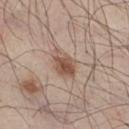{"biopsy_status": "not biopsied; imaged during a skin examination", "lighting": "white-light", "lesion_size": {"long_diameter_mm_approx": 3.5}, "image": {"source": "total-body photography crop", "field_of_view_mm": 15}, "site": "right thigh", "automated_metrics": {"area_mm2_approx": 7.0, "eccentricity": 0.7}, "patient": {"sex": "male", "age_approx": 45}}